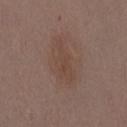follow-up: catalogued during a skin exam; not biopsied
diameter: about 5 mm
patient: female, aged approximately 30
TBP lesion metrics: an area of roughly 9 mm², an eccentricity of roughly 0.9, and a shape-asymmetry score of about 0.3 (0 = symmetric); about 5 CIELAB-L* units darker than the surrounding skin and a normalized lesion–skin contrast near 5; a nevus-likeness score of about 0/100 and a detector confidence of about 100 out of 100 that the crop contains a lesion
anatomic site: the lower back
acquisition: ~15 mm tile from a whole-body skin photo